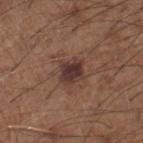biopsy status: total-body-photography surveillance lesion; no biopsy | size: about 3 mm | body site: the leg | tile lighting: white-light illumination | subject: male, aged around 60 | acquisition: total-body-photography crop, ~15 mm field of view.